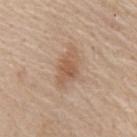Image and clinical context:
A region of skin cropped from a whole-body photographic capture, roughly 15 mm wide. The lesion is on the mid back. A male subject aged 58 to 62.